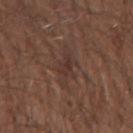{
  "biopsy_status": "not biopsied; imaged during a skin examination",
  "image": {
    "source": "total-body photography crop",
    "field_of_view_mm": 15
  },
  "automated_metrics": {
    "cielab_L": 34,
    "cielab_a": 17,
    "cielab_b": 22,
    "border_irregularity_0_10": 6.5,
    "color_variation_0_10": 3.0,
    "nevus_likeness_0_100": 0,
    "lesion_detection_confidence_0_100": 65
  },
  "lesion_size": {
    "long_diameter_mm_approx": 4.0
  },
  "site": "right forearm",
  "patient": {
    "sex": "male",
    "age_approx": 65
  },
  "lighting": "white-light"
}